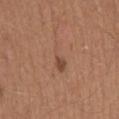<tbp_lesion>
<biopsy_status>not biopsied; imaged during a skin examination</biopsy_status>
<automated_metrics>
  <area_mm2_approx>5.5</area_mm2_approx>
  <eccentricity>0.9</eccentricity>
  <shape_asymmetry>0.4</shape_asymmetry>
</automated_metrics>
<patient>
  <sex>male</sex>
  <age_approx>65</age_approx>
</patient>
<site>mid back</site>
<image>
  <source>total-body photography crop</source>
  <field_of_view_mm>15</field_of_view_mm>
</image>
<lighting>white-light</lighting>
<lesion_size>
  <long_diameter_mm_approx>4.0</long_diameter_mm_approx>
</lesion_size>
</tbp_lesion>